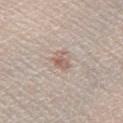{
  "biopsy_status": "not biopsied; imaged during a skin examination",
  "patient": {
    "sex": "male",
    "age_approx": 35
  },
  "lighting": "white-light",
  "site": "right lower leg",
  "automated_metrics": {
    "nevus_likeness_0_100": 35,
    "lesion_detection_confidence_0_100": 95
  },
  "lesion_size": {
    "long_diameter_mm_approx": 2.5
  },
  "image": {
    "source": "total-body photography crop",
    "field_of_view_mm": 15
  }
}